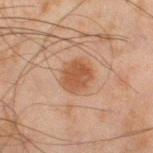Q: Was this lesion biopsied?
A: total-body-photography surveillance lesion; no biopsy
Q: What is the imaging modality?
A: 15 mm crop, total-body photography
Q: What is the anatomic site?
A: the leg
Q: Lesion size?
A: about 3.5 mm
Q: What did automated image analysis measure?
A: an area of roughly 9.5 mm² and a symmetry-axis asymmetry near 0.15; a color-variation rating of about 3/10 and peripheral color asymmetry of about 1
Q: Illumination type?
A: cross-polarized
Q: What are the patient's age and sex?
A: male, aged 43–47